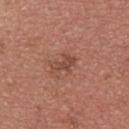This lesion was catalogued during total-body skin photography and was not selected for biopsy. Automated image analysis of the tile measured an area of roughly 4.5 mm², an outline eccentricity of about 0.8 (0 = round, 1 = elongated), and a shape-asymmetry score of about 0.4 (0 = symmetric). And it measured an average lesion color of about L≈47 a*≈24 b*≈28 (CIELAB), roughly 8 lightness units darker than nearby skin, and a normalized lesion–skin contrast near 6. It also reported a border-irregularity rating of about 5/10, a color-variation rating of about 2.5/10, and radial color variation of about 0.5. About 3 mm across. This is a white-light tile. A roughly 15 mm field-of-view crop from a total-body skin photograph. The lesion is located on the upper back. A male subject about 40 years old.